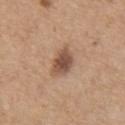Captured during whole-body skin photography for melanoma surveillance; the lesion was not biopsied.
A male patient roughly 70 years of age.
The tile uses white-light illumination.
From the front of the torso.
A lesion tile, about 15 mm wide, cut from a 3D total-body photograph.